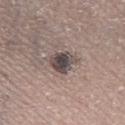workup: catalogued during a skin exam; not biopsied | patient: male, about 65 years old | body site: the leg | TBP lesion metrics: a mean CIELAB color near L≈44 a*≈11 b*≈15, about 15 CIELAB-L* units darker than the surrounding skin, and a normalized lesion–skin contrast near 11.5; a lesion-detection confidence of about 95/100 | image: 15 mm crop, total-body photography | diameter: ~3 mm (longest diameter).